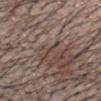| key | value |
|---|---|
| biopsy status | total-body-photography surveillance lesion; no biopsy |
| location | the head or neck |
| patient | male, roughly 40 years of age |
| image-analysis metrics | an outline eccentricity of about 0.7 (0 = round, 1 = elongated); a border-irregularity rating of about 3/10 and peripheral color asymmetry of about 0 |
| tile lighting | white-light |
| size | ≈1 mm |
| imaging modality | 15 mm crop, total-body photography |